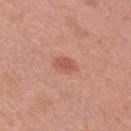Located on the right upper arm. Approximately 3 mm at its widest. A 15 mm crop from a total-body photograph taken for skin-cancer surveillance. The tile uses white-light illumination. A female subject, aged 38–42.